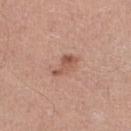{"image": {"source": "total-body photography crop", "field_of_view_mm": 15}, "site": "right lower leg", "patient": {"sex": "male", "age_approx": 60}, "lighting": "white-light"}